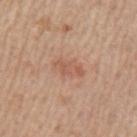This lesion was catalogued during total-body skin photography and was not selected for biopsy. The recorded lesion diameter is about 4 mm. Automated tile analysis of the lesion measured a lesion area of about 7 mm² and a symmetry-axis asymmetry near 0.2. The software also gave an average lesion color of about L≈58 a*≈22 b*≈30 (CIELAB), a lesion–skin lightness drop of about 7, and a normalized lesion–skin contrast near 5. The analysis additionally found a classifier nevus-likeness of about 5/100 and a detector confidence of about 100 out of 100 that the crop contains a lesion. The subject is a male about 75 years old. A roughly 15 mm field-of-view crop from a total-body skin photograph. This is a white-light tile. On the left upper arm.